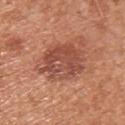The total-body-photography lesion software estimated an area of roughly 19 mm², a shape eccentricity near 0.65, and a symmetry-axis asymmetry near 0.2. It also reported a nevus-likeness score of about 60/100 and lesion-presence confidence of about 100/100.
A lesion tile, about 15 mm wide, cut from a 3D total-body photograph.
The recorded lesion diameter is about 6 mm.
A male patient, approximately 30 years of age.
From the upper back.